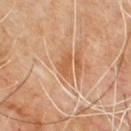Captured during whole-body skin photography for melanoma surveillance; the lesion was not biopsied. About 3 mm across. The total-body-photography lesion software estimated a mean CIELAB color near L≈55 a*≈23 b*≈37 and a lesion–skin lightness drop of about 8. And it measured border irregularity of about 3.5 on a 0–10 scale and a within-lesion color-variation index near 1/10. It also reported a classifier nevus-likeness of about 0/100. Cropped from a whole-body photographic skin survey; the tile spans about 15 mm. Captured under cross-polarized illumination. The lesion is on the upper back. A male subject about 60 years old.